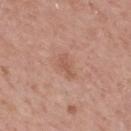| feature | finding |
|---|---|
| biopsy status | no biopsy performed (imaged during a skin exam) |
| patient | male, in their mid- to late 60s |
| imaging modality | 15 mm crop, total-body photography |
| size | about 3 mm |
| location | the upper back |
| lighting | white-light |